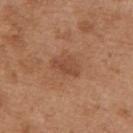{"biopsy_status": "not biopsied; imaged during a skin examination", "lighting": "white-light", "lesion_size": {"long_diameter_mm_approx": 3.5}, "site": "mid back", "patient": {"sex": "female", "age_approx": 40}, "image": {"source": "total-body photography crop", "field_of_view_mm": 15}}